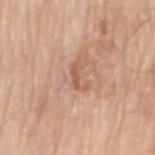Acquisition and patient details: A male subject, approximately 80 years of age. A region of skin cropped from a whole-body photographic capture, roughly 15 mm wide. From the left arm.